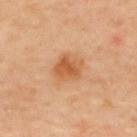notes: imaged on a skin check; not biopsied
patient: male, in their mid- to late 60s
illumination: cross-polarized
lesion diameter: ≈3.5 mm
site: the upper back
automated metrics: a nevus-likeness score of about 95/100 and a detector confidence of about 100 out of 100 that the crop contains a lesion
image source: ~15 mm crop, total-body skin-cancer survey